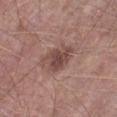Assessment: The lesion was photographed on a routine skin check and not biopsied; there is no pathology result. Clinical summary: The lesion-visualizer software estimated an area of roughly 9 mm², an outline eccentricity of about 0.65 (0 = round, 1 = elongated), and a symmetry-axis asymmetry near 0.3. It also reported a lesion color around L≈46 a*≈20 b*≈22 in CIELAB, about 10 CIELAB-L* units darker than the surrounding skin, and a normalized lesion–skin contrast near 8. The analysis additionally found a border-irregularity rating of about 3/10, a within-lesion color-variation index near 3.5/10, and a peripheral color-asymmetry measure near 1. And it measured a nevus-likeness score of about 30/100 and a detector confidence of about 100 out of 100 that the crop contains a lesion. A male patient about 55 years old. Cropped from a total-body skin-imaging series; the visible field is about 15 mm. Imaged with white-light lighting. Approximately 4 mm at its widest. Located on the left lower leg.